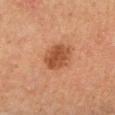Clinical impression:
No biopsy was performed on this lesion — it was imaged during a full skin examination and was not determined to be concerning.
Background:
On the right lower leg. This is a cross-polarized tile. A male patient approximately 65 years of age. Cropped from a whole-body photographic skin survey; the tile spans about 15 mm.